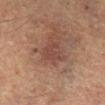Notes:
* workup — no biopsy performed (imaged during a skin exam)
* TBP lesion metrics — a lesion color around L≈37 a*≈19 b*≈22 in CIELAB, about 5 CIELAB-L* units darker than the surrounding skin, and a lesion-to-skin contrast of about 5 (normalized; higher = more distinct)
* acquisition — 15 mm crop, total-body photography
* size — about 3 mm
* patient — male, roughly 55 years of age
* anatomic site — the left lower leg
* illumination — cross-polarized